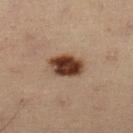biopsy status = catalogued during a skin exam; not biopsied | tile lighting = cross-polarized illumination | site = the left lower leg | patient = male, aged 53 to 57 | lesion size = about 4 mm | imaging modality = ~15 mm crop, total-body skin-cancer survey.